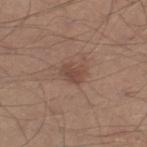The lesion is located on the right thigh. Approximately 3 mm at its widest. Automated image analysis of the tile measured a mean CIELAB color near L≈46 a*≈18 b*≈26 and roughly 8 lightness units darker than nearby skin. The analysis additionally found a border-irregularity index near 2.5/10, a within-lesion color-variation index near 2.5/10, and a peripheral color-asymmetry measure near 1. The analysis additionally found a classifier nevus-likeness of about 15/100. This image is a 15 mm lesion crop taken from a total-body photograph. A male subject aged 28 to 32. Captured under white-light illumination.